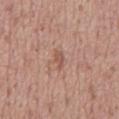Q: Was a biopsy performed?
A: total-body-photography surveillance lesion; no biopsy
Q: What are the patient's age and sex?
A: male, approximately 70 years of age
Q: Illumination type?
A: white-light
Q: What did automated image analysis measure?
A: a lesion color around L≈54 a*≈22 b*≈27 in CIELAB and a normalized lesion–skin contrast near 6; a border-irregularity index near 3.5/10, a color-variation rating of about 0.5/10, and a peripheral color-asymmetry measure near 0; a classifier nevus-likeness of about 0/100 and lesion-presence confidence of about 100/100
Q: What kind of image is this?
A: ~15 mm crop, total-body skin-cancer survey
Q: Where on the body is the lesion?
A: the mid back
Q: How large is the lesion?
A: about 2.5 mm